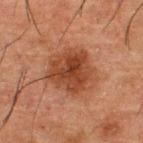| field | value |
|---|---|
| workup | catalogued during a skin exam; not biopsied |
| tile lighting | cross-polarized |
| subject | male, in their 50s |
| diameter | ~5 mm (longest diameter) |
| image | ~15 mm tile from a whole-body skin photo |
| location | the upper back |